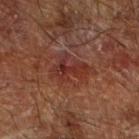| key | value |
|---|---|
| follow-up | imaged on a skin check; not biopsied |
| anatomic site | the right forearm |
| image source | ~15 mm tile from a whole-body skin photo |
| lighting | cross-polarized illumination |
| patient | male, approximately 65 years of age |
| automated metrics | a border-irregularity rating of about 7/10, a color-variation rating of about 4/10, and radial color variation of about 1 |